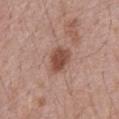Recorded during total-body skin imaging; not selected for excision or biopsy. A close-up tile cropped from a whole-body skin photograph, about 15 mm across. The lesion's longest dimension is about 4 mm. A male patient aged 68 to 72. From the front of the torso. This is a white-light tile. The lesion-visualizer software estimated a mean CIELAB color near L≈49 a*≈22 b*≈27 and a normalized border contrast of about 9. The analysis additionally found a border-irregularity index near 2/10, internal color variation of about 3 on a 0–10 scale, and a peripheral color-asymmetry measure near 1. It also reported a nevus-likeness score of about 80/100 and a detector confidence of about 100 out of 100 that the crop contains a lesion.